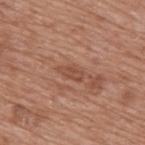This lesion was catalogued during total-body skin photography and was not selected for biopsy. A male patient, approximately 75 years of age. Captured under white-light illumination. The lesion's longest dimension is about 3 mm. A roughly 15 mm field-of-view crop from a total-body skin photograph. From the back.